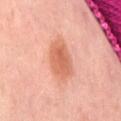– body site: the abdomen
– image source: ~15 mm crop, total-body skin-cancer survey
– lesion diameter: about 4.5 mm
– illumination: cross-polarized
– subject: female, about 50 years old